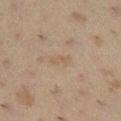This lesion was catalogued during total-body skin photography and was not selected for biopsy. An algorithmic analysis of the crop reported a classifier nevus-likeness of about 0/100 and a detector confidence of about 100 out of 100 that the crop contains a lesion. The tile uses cross-polarized illumination. A 15 mm crop from a total-body photograph taken for skin-cancer surveillance. A female subject about 40 years old. The lesion is on the leg. About 2.5 mm across.